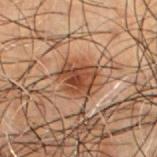Notes:
- biopsy status · no biopsy performed (imaged during a skin exam)
- image · ~15 mm crop, total-body skin-cancer survey
- illumination · cross-polarized
- location · the chest
- patient · male, about 50 years old
- lesion diameter · about 4 mm